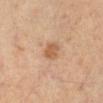| feature | finding |
|---|---|
| location | the left leg |
| TBP lesion metrics | an average lesion color of about L≈55 a*≈20 b*≈34 (CIELAB) and roughly 9 lightness units darker than nearby skin; a border-irregularity rating of about 1.5/10 |
| illumination | cross-polarized illumination |
| lesion diameter | about 2.5 mm |
| image | total-body-photography crop, ~15 mm field of view |
| subject | female, in their mid- to late 60s |